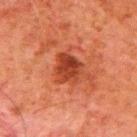follow-up — catalogued during a skin exam; not biopsied | subject — male, aged 78 to 82 | lighting — cross-polarized | image — total-body-photography crop, ~15 mm field of view | location — the back.